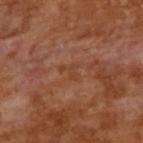Case summary:
- workup: no biopsy performed (imaged during a skin exam)